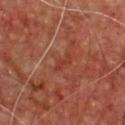Part of a total-body skin-imaging series; this lesion was reviewed on a skin check and was not flagged for biopsy. Cropped from a whole-body photographic skin survey; the tile spans about 15 mm. On the chest. A male subject, roughly 65 years of age. Imaged with cross-polarized lighting. About 2.5 mm across.